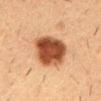{"biopsy_status": "not biopsied; imaged during a skin examination"}